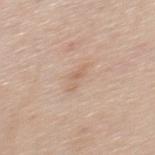Q: Is there a histopathology result?
A: imaged on a skin check; not biopsied
Q: How was the tile lit?
A: white-light
Q: Patient demographics?
A: male, in their mid- to late 30s
Q: Lesion location?
A: the mid back
Q: How was this image acquired?
A: ~15 mm tile from a whole-body skin photo
Q: What is the lesion's diameter?
A: ≈3 mm